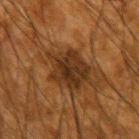Impression: The lesion was tiled from a total-body skin photograph and was not biopsied. Acquisition and patient details: A roughly 15 mm field-of-view crop from a total-body skin photograph. On the arm. The patient is a male aged 63 to 67.